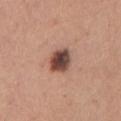Captured during whole-body skin photography for melanoma surveillance; the lesion was not biopsied. The tile uses white-light illumination. The patient is a male aged 43 to 47. This image is a 15 mm lesion crop taken from a total-body photograph. The lesion is located on the chest.